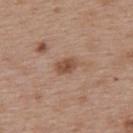Captured during whole-body skin photography for melanoma surveillance; the lesion was not biopsied.
A 15 mm close-up tile from a total-body photography series done for melanoma screening.
Captured under white-light illumination.
The recorded lesion diameter is about 3 mm.
On the upper back.
A female subject, about 40 years old.